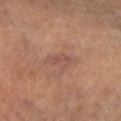<lesion>
<biopsy_status>not biopsied; imaged during a skin examination</biopsy_status>
<site>left lower leg</site>
<lighting>cross-polarized</lighting>
<patient>
  <sex>female</sex>
  <age_approx>65</age_approx>
</patient>
<lesion_size>
  <long_diameter_mm_approx>3.5</long_diameter_mm_approx>
</lesion_size>
<image>
  <source>total-body photography crop</source>
  <field_of_view_mm>15</field_of_view_mm>
</image>
</lesion>